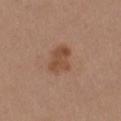Q: Was this lesion biopsied?
A: no biopsy performed (imaged during a skin exam)
Q: What are the patient's age and sex?
A: female, aged approximately 40
Q: What is the anatomic site?
A: the right upper arm
Q: How large is the lesion?
A: ≈4 mm
Q: Illumination type?
A: white-light
Q: How was this image acquired?
A: 15 mm crop, total-body photography